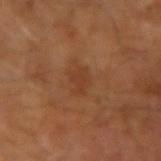<record>
  <biopsy_status>not biopsied; imaged during a skin examination</biopsy_status>
  <patient>
    <sex>male</sex>
    <age_approx>65</age_approx>
  </patient>
  <image>
    <source>total-body photography crop</source>
    <field_of_view_mm>15</field_of_view_mm>
  </image>
  <lighting>cross-polarized</lighting>
  <lesion_size>
    <long_diameter_mm_approx>2.5</long_diameter_mm_approx>
  </lesion_size>
</record>